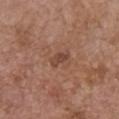Impression:
The lesion was photographed on a routine skin check and not biopsied; there is no pathology result.
Background:
The recorded lesion diameter is about 2.5 mm. A close-up tile cropped from a whole-body skin photograph, about 15 mm across. From the chest. The patient is a female approximately 65 years of age.